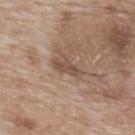biopsy status: imaged on a skin check; not biopsied | site: the upper back | lesion diameter: about 3 mm | image: ~15 mm tile from a whole-body skin photo | subject: female, aged around 75.